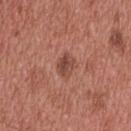workup: catalogued during a skin exam; not biopsied
body site: the head or neck
size: about 3 mm
imaging modality: ~15 mm tile from a whole-body skin photo
subject: male, aged approximately 55